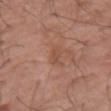This lesion was catalogued during total-body skin photography and was not selected for biopsy. Located on the right upper arm. Longest diameter approximately 3 mm. A male patient aged 68–72. A 15 mm close-up extracted from a 3D total-body photography capture.